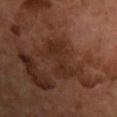follow-up = imaged on a skin check; not biopsied
location = the front of the torso
patient = male, approximately 65 years of age
acquisition = 15 mm crop, total-body photography